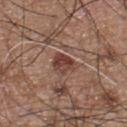{"biopsy_status": "not biopsied; imaged during a skin examination", "patient": {"sex": "male", "age_approx": 55}, "lighting": "white-light", "lesion_size": {"long_diameter_mm_approx": 3.0}, "automated_metrics": {"cielab_L": 41, "cielab_a": 22, "cielab_b": 25, "vs_skin_darker_L": 11.0, "border_irregularity_0_10": 2.5, "color_variation_0_10": 4.0, "peripheral_color_asymmetry": 1.5}, "image": {"source": "total-body photography crop", "field_of_view_mm": 15}, "site": "chest"}